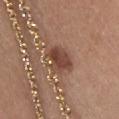The lesion was tiled from a total-body skin photograph and was not biopsied.
The tile uses white-light illumination.
About 3.5 mm across.
The patient is a female in their 40s.
A roughly 15 mm field-of-view crop from a total-body skin photograph.
Located on the chest.